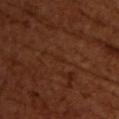Q: Was this lesion biopsied?
A: imaged on a skin check; not biopsied
Q: How large is the lesion?
A: ~1 mm (longest diameter)
Q: What did automated image analysis measure?
A: an area of roughly 0.5 mm², an eccentricity of roughly 0.85, and a shape-asymmetry score of about 0.4 (0 = symmetric); an average lesion color of about L≈26 a*≈22 b*≈28 (CIELAB), a lesion–skin lightness drop of about 4, and a lesion-to-skin contrast of about 4 (normalized; higher = more distinct); a border-irregularity rating of about 3/10, internal color variation of about 0 on a 0–10 scale, and peripheral color asymmetry of about 0
Q: What lighting was used for the tile?
A: cross-polarized illumination
Q: What are the patient's age and sex?
A: female, in their mid-50s
Q: Lesion location?
A: the upper back
Q: What is the imaging modality?
A: ~15 mm tile from a whole-body skin photo